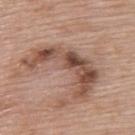Clinical impression: The lesion was photographed on a routine skin check and not biopsied; there is no pathology result. Context: The patient is a female aged 58 to 62. This is a white-light tile. Longest diameter approximately 8.5 mm. From the upper back. A roughly 15 mm field-of-view crop from a total-body skin photograph.